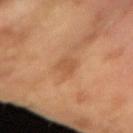Impression:
Captured during whole-body skin photography for melanoma surveillance; the lesion was not biopsied.
Clinical summary:
Captured under cross-polarized illumination. This image is a 15 mm lesion crop taken from a total-body photograph. A male patient, aged 63 to 67. The lesion-visualizer software estimated a lesion area of about 4.5 mm² and a shape-asymmetry score of about 0.25 (0 = symmetric). And it measured a mean CIELAB color near L≈52 a*≈22 b*≈36 and a normalized lesion–skin contrast near 5.5. It also reported an automated nevus-likeness rating near 0 out of 100 and lesion-presence confidence of about 100/100.